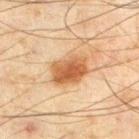<case>
  <biopsy_status>not biopsied; imaged during a skin examination</biopsy_status>
  <site>left thigh</site>
  <image>
    <source>total-body photography crop</source>
    <field_of_view_mm>15</field_of_view_mm>
  </image>
  <patient>
    <sex>male</sex>
    <age_approx>45</age_approx>
  </patient>
  <automated_metrics>
    <vs_skin_contrast_norm>9.0</vs_skin_contrast_norm>
  </automated_metrics>
  <lesion_size>
    <long_diameter_mm_approx>5.0</long_diameter_mm_approx>
  </lesion_size>
</case>